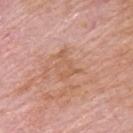Notes:
* diameter — ≈3.5 mm
* location — the back
* imaging modality — ~15 mm tile from a whole-body skin photo
* subject — male, approximately 65 years of age
* automated lesion analysis — an area of roughly 5 mm² and a symmetry-axis asymmetry near 0.65; a normalized lesion–skin contrast near 5.5; border irregularity of about 9.5 on a 0–10 scale and a peripheral color-asymmetry measure near 0